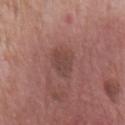Q: Is there a histopathology result?
A: catalogued during a skin exam; not biopsied
Q: What is the anatomic site?
A: the front of the torso
Q: What is the imaging modality?
A: 15 mm crop, total-body photography
Q: What is the lesion's diameter?
A: ~3 mm (longest diameter)
Q: Automated lesion metrics?
A: border irregularity of about 2.5 on a 0–10 scale, a within-lesion color-variation index near 2.5/10, and radial color variation of about 1
Q: What are the patient's age and sex?
A: male, in their 80s